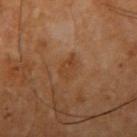Imaged during a routine full-body skin examination; the lesion was not biopsied and no histopathology is available. Captured under cross-polarized illumination. The subject is a male in their 60s. The lesion is located on the right upper arm. The lesion-visualizer software estimated a lesion color around L≈40 a*≈21 b*≈33 in CIELAB, about 5 CIELAB-L* units darker than the surrounding skin, and a lesion-to-skin contrast of about 5 (normalized; higher = more distinct). The analysis additionally found a border-irregularity index near 3/10, internal color variation of about 2 on a 0–10 scale, and peripheral color asymmetry of about 0.5. A 15 mm close-up extracted from a 3D total-body photography capture. The recorded lesion diameter is about 3 mm.